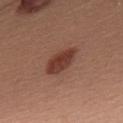{
  "biopsy_status": "not biopsied; imaged during a skin examination",
  "image": {
    "source": "total-body photography crop",
    "field_of_view_mm": 15
  },
  "patient": {
    "sex": "male",
    "age_approx": 55
  },
  "site": "upper back",
  "lesion_size": {
    "long_diameter_mm_approx": 4.5
  },
  "automated_metrics": {
    "border_irregularity_0_10": 2.0,
    "color_variation_0_10": 3.0,
    "peripheral_color_asymmetry": 1.0,
    "nevus_likeness_0_100": 100,
    "lesion_detection_confidence_0_100": 100
  }
}